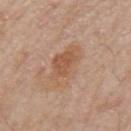Captured during whole-body skin photography for melanoma surveillance; the lesion was not biopsied. Imaged with white-light lighting. The lesion's longest dimension is about 5 mm. The total-body-photography lesion software estimated an area of roughly 12 mm² and a shape eccentricity near 0.8. Located on the arm. A male subject, aged approximately 80. This image is a 15 mm lesion crop taken from a total-body photograph.